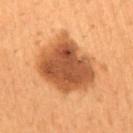Findings:
* notes: no biopsy performed (imaged during a skin exam)
* lighting: cross-polarized illumination
* patient: male, aged around 50
* body site: the mid back
* image source: ~15 mm crop, total-body skin-cancer survey
* diameter: ~6.5 mm (longest diameter)
* image-analysis metrics: a lesion color around L≈42 a*≈22 b*≈33 in CIELAB and a lesion–skin lightness drop of about 14; an automated nevus-likeness rating near 65 out of 100 and a lesion-detection confidence of about 100/100